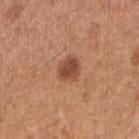notes — catalogued during a skin exam; not biopsied
acquisition — total-body-photography crop, ~15 mm field of view
tile lighting — white-light illumination
lesion size — about 3 mm
automated metrics — an area of roughly 6 mm²; an automated nevus-likeness rating near 95 out of 100 and a detector confidence of about 100 out of 100 that the crop contains a lesion
subject — female, aged approximately 30
anatomic site — the arm